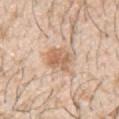<case>
<biopsy_status>not biopsied; imaged during a skin examination</biopsy_status>
<site>left upper arm</site>
<patient>
  <sex>male</sex>
  <age_approx>30</age_approx>
</patient>
<image>
  <source>total-body photography crop</source>
  <field_of_view_mm>15</field_of_view_mm>
</image>
</case>